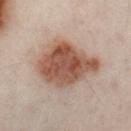Clinical impression: Imaged during a routine full-body skin examination; the lesion was not biopsied and no histopathology is available. Image and clinical context: Measured at roughly 8.5 mm in maximum diameter. A male patient aged around 50. This image is a 15 mm lesion crop taken from a total-body photograph. Automated tile analysis of the lesion measured a footprint of about 27 mm², an outline eccentricity of about 0.8 (0 = round, 1 = elongated), and a shape-asymmetry score of about 0.25 (0 = symmetric). And it measured an average lesion color of about L≈42 a*≈17 b*≈23 (CIELAB), roughly 13 lightness units darker than nearby skin, and a lesion-to-skin contrast of about 10.5 (normalized; higher = more distinct). And it measured a classifier nevus-likeness of about 100/100 and lesion-presence confidence of about 100/100. On the left leg. Captured under cross-polarized illumination.